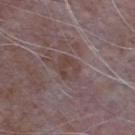Q: Is there a histopathology result?
A: total-body-photography surveillance lesion; no biopsy
Q: Where on the body is the lesion?
A: the chest
Q: What are the patient's age and sex?
A: male, aged 63–67
Q: What kind of image is this?
A: 15 mm crop, total-body photography
Q: What is the lesion's diameter?
A: ≈3 mm
Q: What lighting was used for the tile?
A: white-light
Q: Automated lesion metrics?
A: a lesion area of about 6 mm², a shape eccentricity near 0.55, and a shape-asymmetry score of about 0.2 (0 = symmetric); a classifier nevus-likeness of about 0/100 and lesion-presence confidence of about 85/100